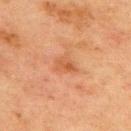biopsy status — no biopsy performed (imaged during a skin exam); imaging modality — 15 mm crop, total-body photography; site — the upper back; subject — male, roughly 70 years of age.